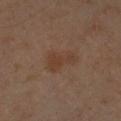Q: What is the imaging modality?
A: ~15 mm crop, total-body skin-cancer survey
Q: Lesion location?
A: the left forearm
Q: Who is the patient?
A: male, roughly 60 years of age